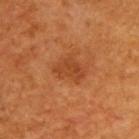Clinical impression:
This lesion was catalogued during total-body skin photography and was not selected for biopsy.
Context:
From the upper back. A male patient, aged 58–62. Imaged with cross-polarized lighting. Cropped from a total-body skin-imaging series; the visible field is about 15 mm.